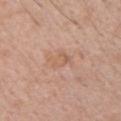Located on the chest.
The lesion-visualizer software estimated a mean CIELAB color near L≈60 a*≈20 b*≈31, roughly 6 lightness units darker than nearby skin, and a normalized lesion–skin contrast near 5. The analysis additionally found an automated nevus-likeness rating near 0 out of 100 and a lesion-detection confidence of about 100/100.
Cropped from a whole-body photographic skin survey; the tile spans about 15 mm.
This is a white-light tile.
The lesion's longest dimension is about 3 mm.
A male patient aged around 65.